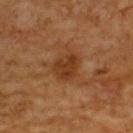The lesion was photographed on a routine skin check and not biopsied; there is no pathology result. The lesion's longest dimension is about 3.5 mm. An algorithmic analysis of the crop reported a footprint of about 8.5 mm², an outline eccentricity of about 0.55 (0 = round, 1 = elongated), and two-axis asymmetry of about 0.25. The software also gave a border-irregularity rating of about 2.5/10, internal color variation of about 3 on a 0–10 scale, and peripheral color asymmetry of about 1. On the upper back. A male subject aged around 60. A region of skin cropped from a whole-body photographic capture, roughly 15 mm wide. Imaged with cross-polarized lighting.